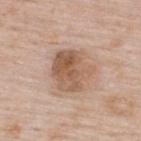On the upper back.
The tile uses white-light illumination.
A female patient roughly 50 years of age.
This image is a 15 mm lesion crop taken from a total-body photograph.
The total-body-photography lesion software estimated a lesion color around L≈57 a*≈18 b*≈30 in CIELAB and a lesion-to-skin contrast of about 7.5 (normalized; higher = more distinct). The software also gave a within-lesion color-variation index near 6.5/10.
Approximately 5 mm at its widest.